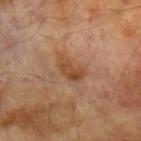The lesion was tiled from a total-body skin photograph and was not biopsied. Cropped from a total-body skin-imaging series; the visible field is about 15 mm. The recorded lesion diameter is about 3.5 mm. A male patient, aged 68 to 72. On the left upper arm.